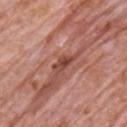{
  "biopsy_status": "not biopsied; imaged during a skin examination",
  "automated_metrics": {
    "area_mm2_approx": 3.0,
    "eccentricity": 0.85,
    "shape_asymmetry": 0.3,
    "cielab_L": 45,
    "cielab_a": 26,
    "cielab_b": 28
  },
  "lesion_size": {
    "long_diameter_mm_approx": 2.5
  },
  "image": {
    "source": "total-body photography crop",
    "field_of_view_mm": 15
  },
  "site": "front of the torso",
  "patient": {
    "sex": "male",
    "age_approx": 80
  },
  "lighting": "white-light"
}